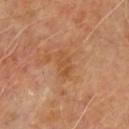No biopsy was performed on this lesion — it was imaged during a full skin examination and was not determined to be concerning. The lesion is located on the upper back. The tile uses cross-polarized illumination. A male subject, aged 58–62. About 3.5 mm across. Cropped from a whole-body photographic skin survey; the tile spans about 15 mm.